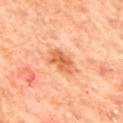notes=total-body-photography surveillance lesion; no biopsy
lesion diameter=≈4 mm
body site=the mid back
illumination=cross-polarized illumination
image source=total-body-photography crop, ~15 mm field of view
patient=male, aged approximately 65